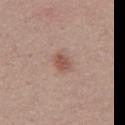Q: Was a biopsy performed?
A: total-body-photography surveillance lesion; no biopsy
Q: Where on the body is the lesion?
A: the mid back
Q: What is the imaging modality?
A: 15 mm crop, total-body photography
Q: What is the lesion's diameter?
A: ~2.5 mm (longest diameter)
Q: What are the patient's age and sex?
A: male, approximately 55 years of age
Q: How was the tile lit?
A: white-light